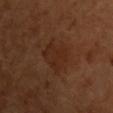biopsy_status: not biopsied; imaged during a skin examination
image:
  source: total-body photography crop
  field_of_view_mm: 15
site: front of the torso
patient:
  sex: male
  age_approx: 50